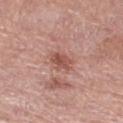{"biopsy_status": "not biopsied; imaged during a skin examination", "lesion_size": {"long_diameter_mm_approx": 3.0}, "lighting": "white-light", "patient": {"sex": "female", "age_approx": 55}, "image": {"source": "total-body photography crop", "field_of_view_mm": 15}, "site": "leg"}